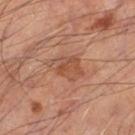follow-up: imaged on a skin check; not biopsied | imaging modality: 15 mm crop, total-body photography | TBP lesion metrics: an area of roughly 8 mm², an eccentricity of roughly 0.75, and a shape-asymmetry score of about 0.3 (0 = symmetric); an automated nevus-likeness rating near 5 out of 100 and a detector confidence of about 100 out of 100 that the crop contains a lesion | location: the left thigh | subject: male, in their mid-50s.